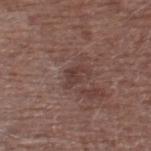Q: Was a biopsy performed?
A: catalogued during a skin exam; not biopsied
Q: Illumination type?
A: white-light illumination
Q: What did automated image analysis measure?
A: a border-irregularity index near 6/10, a color-variation rating of about 2.5/10, and radial color variation of about 1
Q: What are the patient's age and sex?
A: male, about 70 years old
Q: What is the anatomic site?
A: the right lower leg
Q: How large is the lesion?
A: ≈3 mm
Q: What is the imaging modality?
A: ~15 mm tile from a whole-body skin photo